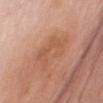Impression: This lesion was catalogued during total-body skin photography and was not selected for biopsy. Clinical summary: From the chest. The lesion's longest dimension is about 5.5 mm. Captured under white-light illumination. A female subject, aged around 65. A close-up tile cropped from a whole-body skin photograph, about 15 mm across.